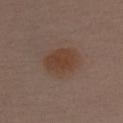workup: total-body-photography surveillance lesion; no biopsy | lesion diameter: about 4 mm | tile lighting: white-light | imaging modality: total-body-photography crop, ~15 mm field of view | anatomic site: the right upper arm | patient: male, aged around 40.